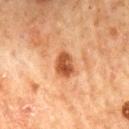Notes:
* notes · no biopsy performed (imaged during a skin exam)
* anatomic site · the mid back
* lighting · cross-polarized illumination
* automated lesion analysis · a footprint of about 6 mm², an outline eccentricity of about 0.7 (0 = round, 1 = elongated), and two-axis asymmetry of about 0.15; a border-irregularity index near 1.5/10 and internal color variation of about 4.5 on a 0–10 scale
* image source · 15 mm crop, total-body photography
* diameter · ~3.5 mm (longest diameter)
* patient · male, in their mid-60s